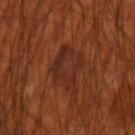{"biopsy_status": "not biopsied; imaged during a skin examination", "site": "arm", "patient": {"sex": "male", "age_approx": 70}, "image": {"source": "total-body photography crop", "field_of_view_mm": 15}, "lesion_size": {"long_diameter_mm_approx": 4.5}, "lighting": "cross-polarized", "automated_metrics": {"eccentricity": 0.55, "shape_asymmetry": 0.2, "lesion_detection_confidence_0_100": 95}}